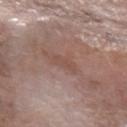Case summary:
– follow-up · no biopsy performed (imaged during a skin exam)
– anatomic site · the left forearm
– imaging modality · 15 mm crop, total-body photography
– patient · male, aged approximately 60
– diameter · ≈2.5 mm
– illumination · white-light